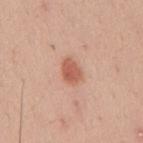The lesion was photographed on a routine skin check and not biopsied; there is no pathology result. A male subject, aged approximately 30. Cropped from a whole-body photographic skin survey; the tile spans about 15 mm. From the mid back.